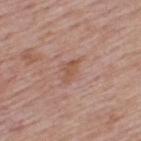Clinical impression: The lesion was tiled from a total-body skin photograph and was not biopsied. Image and clinical context: From the upper back. This is a white-light tile. The lesion-visualizer software estimated an area of roughly 3 mm² and a shape eccentricity near 0.9. The software also gave an average lesion color of about L≈53 a*≈21 b*≈29 (CIELAB). A male patient aged 63 to 67. Measured at roughly 3 mm in maximum diameter. A close-up tile cropped from a whole-body skin photograph, about 15 mm across.